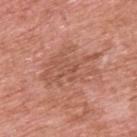This lesion was catalogued during total-body skin photography and was not selected for biopsy.
The subject is a male aged around 70.
Approximately 7 mm at its widest.
Captured under white-light illumination.
The lesion is located on the upper back.
This image is a 15 mm lesion crop taken from a total-body photograph.